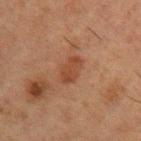Impression:
Part of a total-body skin-imaging series; this lesion was reviewed on a skin check and was not flagged for biopsy.
Background:
The lesion's longest dimension is about 3.5 mm. A 15 mm close-up tile from a total-body photography series done for melanoma screening. Imaged with cross-polarized lighting. A male patient, roughly 50 years of age. From the left upper arm. An algorithmic analysis of the crop reported a border-irregularity rating of about 2.5/10, a within-lesion color-variation index near 1.5/10, and a peripheral color-asymmetry measure near 0.5. The software also gave a detector confidence of about 100 out of 100 that the crop contains a lesion.